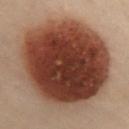<record>
<biopsy_status>not biopsied; imaged during a skin examination</biopsy_status>
<lighting>cross-polarized</lighting>
<lesion_size>
  <long_diameter_mm_approx>12.5</long_diameter_mm_approx>
</lesion_size>
<site>chest</site>
<automated_metrics>
  <area_mm2_approx>90.0</area_mm2_approx>
  <cielab_L>34</cielab_L>
  <cielab_a>20</cielab_a>
  <cielab_b>25</cielab_b>
  <vs_skin_darker_L>19.0</vs_skin_darker_L>
  <vs_skin_contrast_norm>15.0</vs_skin_contrast_norm>
  <color_variation_0_10>8.5</color_variation_0_10>
  <nevus_likeness_0_100>100</nevus_likeness_0_100>
</automated_metrics>
<image>
  <source>total-body photography crop</source>
  <field_of_view_mm>15</field_of_view_mm>
</image>
<patient>
  <sex>female</sex>
  <age_approx>60</age_approx>
</patient>
</record>